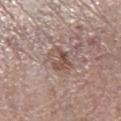biopsy status: total-body-photography surveillance lesion; no biopsy | subject: female, aged approximately 70 | body site: the right lower leg | diameter: ≈3.5 mm | image source: ~15 mm tile from a whole-body skin photo | automated lesion analysis: a lesion area of about 7 mm², an eccentricity of roughly 0.7, and a shape-asymmetry score of about 0.3 (0 = symmetric); a border-irregularity index near 3.5/10 and a within-lesion color-variation index near 5.5/10.